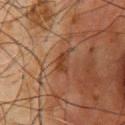biopsy status: catalogued during a skin exam; not biopsied | anatomic site: the chest | size: about 2.5 mm | lighting: cross-polarized illumination | image: ~15 mm tile from a whole-body skin photo | image-analysis metrics: a symmetry-axis asymmetry near 0.35 | subject: male, aged around 60.